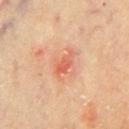notes=total-body-photography surveillance lesion; no biopsy
lighting=cross-polarized illumination
patient=male, about 65 years old
imaging modality=~15 mm crop, total-body skin-cancer survey
body site=the front of the torso
TBP lesion metrics=an area of roughly 4.5 mm² and a symmetry-axis asymmetry near 0.25; a nevus-likeness score of about 0/100
diameter=≈3.5 mm